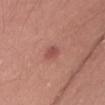notes: catalogued during a skin exam; not biopsied
image: ~15 mm crop, total-body skin-cancer survey
illumination: white-light
lesion size: about 3 mm
patient: male, in their mid- to late 20s
anatomic site: the lower back